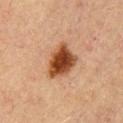Impression:
Imaged during a routine full-body skin examination; the lesion was not biopsied and no histopathology is available.
Background:
An algorithmic analysis of the crop reported a mean CIELAB color near L≈42 a*≈23 b*≈33, a lesion–skin lightness drop of about 16, and a normalized border contrast of about 12.5. The analysis additionally found a classifier nevus-likeness of about 100/100 and a detector confidence of about 100 out of 100 that the crop contains a lesion. Imaged with cross-polarized lighting. The subject is a male roughly 60 years of age. The recorded lesion diameter is about 4.5 mm. Cropped from a total-body skin-imaging series; the visible field is about 15 mm.